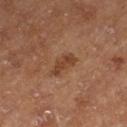notes = catalogued during a skin exam; not biopsied | tile lighting = cross-polarized | lesion size = ≈3.5 mm | subject = male, approximately 65 years of age | imaging modality = ~15 mm crop, total-body skin-cancer survey | site = the left thigh.